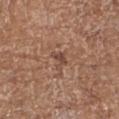Impression:
Part of a total-body skin-imaging series; this lesion was reviewed on a skin check and was not flagged for biopsy.
Clinical summary:
The lesion is located on the left upper arm. A female subject, aged 73–77. A lesion tile, about 15 mm wide, cut from a 3D total-body photograph. Imaged with white-light lighting.